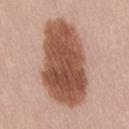{
  "biopsy_status": "not biopsied; imaged during a skin examination",
  "site": "lower back",
  "automated_metrics": {
    "cielab_L": 52,
    "cielab_a": 22,
    "cielab_b": 29,
    "vs_skin_darker_L": 18.0,
    "vs_skin_contrast_norm": 12.0
  },
  "patient": {
    "sex": "female",
    "age_approx": 20
  },
  "image": {
    "source": "total-body photography crop",
    "field_of_view_mm": 15
  },
  "lesion_size": {
    "long_diameter_mm_approx": 10.5
  },
  "lighting": "white-light"
}